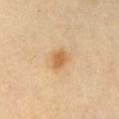Q: How was the tile lit?
A: cross-polarized illumination
Q: What kind of image is this?
A: ~15 mm tile from a whole-body skin photo
Q: Lesion location?
A: the chest
Q: Lesion size?
A: ~3 mm (longest diameter)
Q: What did automated image analysis measure?
A: a footprint of about 4.5 mm², an outline eccentricity of about 0.7 (0 = round, 1 = elongated), and two-axis asymmetry of about 0.2; a lesion color around L≈63 a*≈20 b*≈42 in CIELAB, a lesion–skin lightness drop of about 11, and a normalized border contrast of about 7.5; lesion-presence confidence of about 100/100
Q: Who is the patient?
A: female, approximately 45 years of age